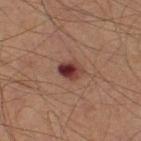Assessment:
Part of a total-body skin-imaging series; this lesion was reviewed on a skin check and was not flagged for biopsy.
Image and clinical context:
A male subject, aged 58 to 62. Longest diameter approximately 2.5 mm. The lesion is on the right thigh. The tile uses cross-polarized illumination. A 15 mm close-up tile from a total-body photography series done for melanoma screening.